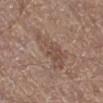Q: Is there a histopathology result?
A: no biopsy performed (imaged during a skin exam)
Q: What is the anatomic site?
A: the right lower leg
Q: Patient demographics?
A: male, aged around 70
Q: How was this image acquired?
A: 15 mm crop, total-body photography